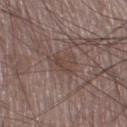The lesion is on the left thigh. The lesion-visualizer software estimated a border-irregularity index near 4/10, internal color variation of about 3 on a 0–10 scale, and a peripheral color-asymmetry measure near 1. A 15 mm close-up extracted from a 3D total-body photography capture. The lesion's longest dimension is about 3 mm. The subject is a male roughly 75 years of age. The tile uses white-light illumination.